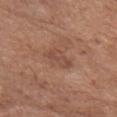biopsy status: total-body-photography surveillance lesion; no biopsy
automated metrics: an area of roughly 5.5 mm², an eccentricity of roughly 0.8, and a shape-asymmetry score of about 0.3 (0 = symmetric); a lesion color around L≈48 a*≈21 b*≈28 in CIELAB and a lesion–skin lightness drop of about 7; lesion-presence confidence of about 100/100
image source: ~15 mm tile from a whole-body skin photo
location: the head or neck
subject: female, aged 73 to 77